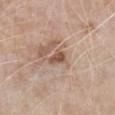  patient:
    sex: male
    age_approx: 80
  lesion_size:
    long_diameter_mm_approx: 2.5
  image:
    source: total-body photography crop
    field_of_view_mm: 15
  automated_metrics:
    cielab_L: 53
    cielab_a: 19
    cielab_b: 27
    vs_skin_darker_L: 11.0
    vs_skin_contrast_norm: 8.0
    border_irregularity_0_10: 3.0
    color_variation_0_10: 2.5
    peripheral_color_asymmetry: 1.0
  lighting: white-light
  site: chest